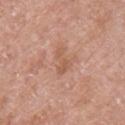A female patient aged approximately 60. Automated image analysis of the tile measured a within-lesion color-variation index near 1/10 and a peripheral color-asymmetry measure near 0.5. And it measured a lesion-detection confidence of about 100/100. Captured under white-light illumination. A close-up tile cropped from a whole-body skin photograph, about 15 mm across. The lesion is located on the chest.